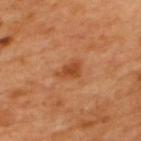No biopsy was performed on this lesion — it was imaged during a full skin examination and was not determined to be concerning.
Imaged with cross-polarized lighting.
The lesion-visualizer software estimated a lesion area of about 4.5 mm², an eccentricity of roughly 0.75, and a symmetry-axis asymmetry near 0.3. The analysis additionally found border irregularity of about 3 on a 0–10 scale, a within-lesion color-variation index near 2.5/10, and peripheral color asymmetry of about 1.
The lesion is on the upper back.
The lesion's longest dimension is about 3 mm.
A male subject, aged 48–52.
A close-up tile cropped from a whole-body skin photograph, about 15 mm across.